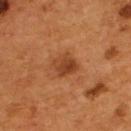* workup — no biopsy performed (imaged during a skin exam)
* subject — male, aged 53 to 57
* tile lighting — cross-polarized illumination
* image source — ~15 mm crop, total-body skin-cancer survey
* location — the upper back